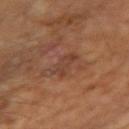Impression: Imaged during a routine full-body skin examination; the lesion was not biopsied and no histopathology is available. Context: The lesion is on the left arm. Imaged with cross-polarized lighting. A 15 mm close-up extracted from a 3D total-body photography capture. Automated tile analysis of the lesion measured a footprint of about 6 mm², a shape eccentricity near 0.8, and two-axis asymmetry of about 0.25. The analysis additionally found a border-irregularity rating of about 3.5/10, internal color variation of about 2.5 on a 0–10 scale, and radial color variation of about 0.5. Measured at roughly 3.5 mm in maximum diameter. A male subject, aged approximately 65.